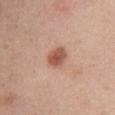No biopsy was performed on this lesion — it was imaged during a full skin examination and was not determined to be concerning. The tile uses white-light illumination. The lesion is on the abdomen. The lesion's longest dimension is about 3 mm. The patient is a female aged 48–52. A 15 mm crop from a total-body photograph taken for skin-cancer surveillance.